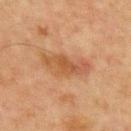Q: Is there a histopathology result?
A: catalogued during a skin exam; not biopsied